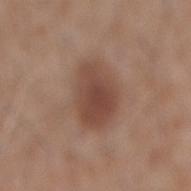No biopsy was performed on this lesion — it was imaged during a full skin examination and was not determined to be concerning.
A close-up tile cropped from a whole-body skin photograph, about 15 mm across.
Approximately 5.5 mm at its widest.
On the mid back.
A male subject in their 60s.
Captured under white-light illumination.